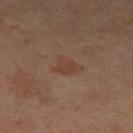<record>
  <biopsy_status>not biopsied; imaged during a skin examination</biopsy_status>
  <automated_metrics>
    <border_irregularity_0_10>4.5</border_irregularity_0_10>
    <color_variation_0_10>1.5</color_variation_0_10>
    <peripheral_color_asymmetry>0.5</peripheral_color_asymmetry>
    <nevus_likeness_0_100>5</nevus_likeness_0_100>
    <lesion_detection_confidence_0_100>100</lesion_detection_confidence_0_100>
  </automated_metrics>
  <lighting>cross-polarized</lighting>
  <patient>
    <sex>female</sex>
    <age_approx>65</age_approx>
  </patient>
  <image>
    <source>total-body photography crop</source>
    <field_of_view_mm>15</field_of_view_mm>
  </image>
  <lesion_size>
    <long_diameter_mm_approx>3.0</long_diameter_mm_approx>
  </lesion_size>
  <site>left thigh</site>
</record>